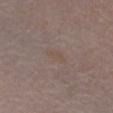{"biopsy_status": "not biopsied; imaged during a skin examination", "patient": {"sex": "female", "age_approx": 80}, "site": "chest", "lighting": "white-light", "image": {"source": "total-body photography crop", "field_of_view_mm": 15}, "lesion_size": {"long_diameter_mm_approx": 3.0}}